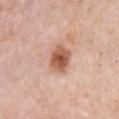{
  "biopsy_status": "not biopsied; imaged during a skin examination",
  "automated_metrics": {
    "area_mm2_approx": 9.0,
    "eccentricity": 0.7,
    "cielab_L": 58,
    "cielab_a": 23,
    "cielab_b": 31,
    "vs_skin_darker_L": 14.0,
    "vs_skin_contrast_norm": 9.5,
    "border_irregularity_0_10": 1.5,
    "color_variation_0_10": 4.5,
    "peripheral_color_asymmetry": 1.0,
    "nevus_likeness_0_100": 85,
    "lesion_detection_confidence_0_100": 100
  },
  "lighting": "white-light",
  "site": "front of the torso",
  "image": {
    "source": "total-body photography crop",
    "field_of_view_mm": 15
  },
  "lesion_size": {
    "long_diameter_mm_approx": 4.0
  },
  "patient": {
    "sex": "female",
    "age_approx": 60
  }
}